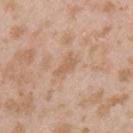Captured during whole-body skin photography for melanoma surveillance; the lesion was not biopsied.
Longest diameter approximately 3 mm.
Imaged with white-light lighting.
The lesion is located on the left upper arm.
A female subject, aged around 25.
Cropped from a whole-body photographic skin survey; the tile spans about 15 mm.
The lesion-visualizer software estimated a lesion color around L≈60 a*≈19 b*≈33 in CIELAB. The analysis additionally found a within-lesion color-variation index near 0.5/10 and a peripheral color-asymmetry measure near 0.